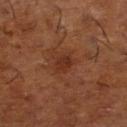Clinical impression: The lesion was photographed on a routine skin check and not biopsied; there is no pathology result. Acquisition and patient details: Captured under cross-polarized illumination. A male subject aged 63–67. Cropped from a total-body skin-imaging series; the visible field is about 15 mm. On the leg.